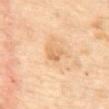<lesion>
  <biopsy_status>not biopsied; imaged during a skin examination</biopsy_status>
  <image>
    <source>total-body photography crop</source>
    <field_of_view_mm>15</field_of_view_mm>
  </image>
  <lighting>cross-polarized</lighting>
  <site>mid back</site>
  <lesion_size>
    <long_diameter_mm_approx>2.5</long_diameter_mm_approx>
  </lesion_size>
  <patient>
    <sex>female</sex>
    <age_approx>60</age_approx>
  </patient>
</lesion>